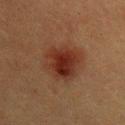Q: Is there a histopathology result?
A: no biopsy performed (imaged during a skin exam)
Q: How was this image acquired?
A: total-body-photography crop, ~15 mm field of view
Q: Automated lesion metrics?
A: an automated nevus-likeness rating near 95 out of 100 and a detector confidence of about 100 out of 100 that the crop contains a lesion
Q: Patient demographics?
A: male, in their 40s
Q: How large is the lesion?
A: ≈5 mm
Q: Lesion location?
A: the left upper arm